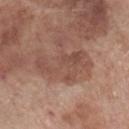notes: imaged on a skin check; not biopsied | patient: male, aged 68 to 72 | tile lighting: white-light illumination | image source: total-body-photography crop, ~15 mm field of view | diameter: ≈7 mm | location: the right lower leg.